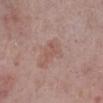This lesion was catalogued during total-body skin photography and was not selected for biopsy.
The lesion-visualizer software estimated a shape eccentricity near 0.8 and a symmetry-axis asymmetry near 0.35. The software also gave a mean CIELAB color near L≈54 a*≈20 b*≈23, about 6 CIELAB-L* units darker than the surrounding skin, and a normalized lesion–skin contrast near 4.5.
Approximately 3 mm at its widest.
The subject is a male roughly 80 years of age.
This image is a 15 mm lesion crop taken from a total-body photograph.
Captured under white-light illumination.
On the right lower leg.